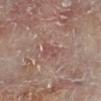Captured during whole-body skin photography for melanoma surveillance; the lesion was not biopsied. The patient is a male roughly 60 years of age. A roughly 15 mm field-of-view crop from a total-body skin photograph. Longest diameter approximately 3 mm. The lesion is located on the right lower leg.